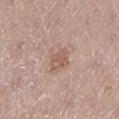Impression:
The lesion was tiled from a total-body skin photograph and was not biopsied.
Acquisition and patient details:
A female patient aged 48–52. A lesion tile, about 15 mm wide, cut from a 3D total-body photograph. This is a white-light tile. Measured at roughly 3.5 mm in maximum diameter. The lesion is on the left lower leg. An algorithmic analysis of the crop reported about 8 CIELAB-L* units darker than the surrounding skin and a normalized border contrast of about 6. The analysis additionally found an automated nevus-likeness rating near 5 out of 100.